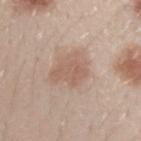A 15 mm crop from a total-body photograph taken for skin-cancer surveillance. On the arm. The subject is a male about 30 years old.